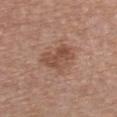Captured during whole-body skin photography for melanoma surveillance; the lesion was not biopsied. From the chest. The lesion's longest dimension is about 4.5 mm. The subject is a female roughly 60 years of age. A 15 mm close-up tile from a total-body photography series done for melanoma screening.